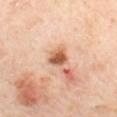Case summary:
• workup — no biopsy performed (imaged during a skin exam)
• size — ≈2.5 mm
• patient — female, aged around 60
• body site — the mid back
• tile lighting — cross-polarized
• imaging modality — ~15 mm crop, total-body skin-cancer survey
• image-analysis metrics — an outline eccentricity of about 0.35 (0 = round, 1 = elongated) and two-axis asymmetry of about 0.25; a nevus-likeness score of about 85/100 and lesion-presence confidence of about 100/100